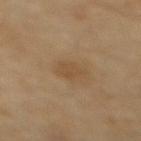A male patient approximately 70 years of age.
A close-up tile cropped from a whole-body skin photograph, about 15 mm across.
The lesion is located on the mid back.
Imaged with cross-polarized lighting.
The lesion's longest dimension is about 3 mm.